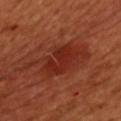follow-up=catalogued during a skin exam; not biopsied | image source=total-body-photography crop, ~15 mm field of view | subject=male, roughly 50 years of age | site=the front of the torso | image-analysis metrics=border irregularity of about 5.5 on a 0–10 scale and a color-variation rating of about 3.5/10; an automated nevus-likeness rating near 60 out of 100 and a detector confidence of about 100 out of 100 that the crop contains a lesion.